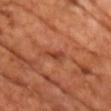The lesion was photographed on a routine skin check and not biopsied; there is no pathology result. This is a cross-polarized tile. The patient is a male about 70 years old. The lesion is on the chest. Approximately 2.5 mm at its widest. An algorithmic analysis of the crop reported a lesion area of about 3 mm². The software also gave a mean CIELAB color near L≈42 a*≈29 b*≈34 and a normalized border contrast of about 6.5. It also reported border irregularity of about 4.5 on a 0–10 scale, a within-lesion color-variation index near 1.5/10, and peripheral color asymmetry of about 0.5. A region of skin cropped from a whole-body photographic capture, roughly 15 mm wide.